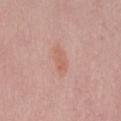Impression: No biopsy was performed on this lesion — it was imaged during a full skin examination and was not determined to be concerning. Context: Cropped from a total-body skin-imaging series; the visible field is about 15 mm. Located on the mid back. A female patient roughly 60 years of age.